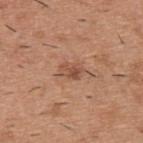Findings:
* follow-up · catalogued during a skin exam; not biopsied
* tile lighting · white-light
* location · the upper back
* automated metrics · an area of roughly 4.5 mm², an outline eccentricity of about 0.75 (0 = round, 1 = elongated), and two-axis asymmetry of about 0.2; a mean CIELAB color near L≈51 a*≈23 b*≈30, about 9 CIELAB-L* units darker than the surrounding skin, and a normalized lesion–skin contrast near 6.5; a color-variation rating of about 4/10 and a peripheral color-asymmetry measure near 1.5; lesion-presence confidence of about 100/100
* patient · male, roughly 40 years of age
* image · total-body-photography crop, ~15 mm field of view